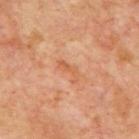Impression:
Imaged during a routine full-body skin examination; the lesion was not biopsied and no histopathology is available.
Context:
The lesion is on the mid back. A patient in their mid- to late 60s. A region of skin cropped from a whole-body photographic capture, roughly 15 mm wide.